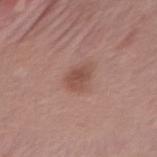Captured during whole-body skin photography for melanoma surveillance; the lesion was not biopsied.